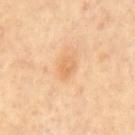Notes:
• workup · no biopsy performed (imaged during a skin exam)
• location · the mid back
• illumination · cross-polarized illumination
• imaging modality · ~15 mm tile from a whole-body skin photo